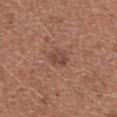image source: total-body-photography crop, ~15 mm field of view
automated metrics: a footprint of about 3.5 mm², a shape eccentricity near 0.7, and a shape-asymmetry score of about 0.25 (0 = symmetric); a border-irregularity rating of about 2.5/10 and a peripheral color-asymmetry measure near 0.5; a classifier nevus-likeness of about 15/100 and a lesion-detection confidence of about 100/100
illumination: white-light
body site: the left upper arm
patient: female, about 35 years old
lesion diameter: ≈2.5 mm